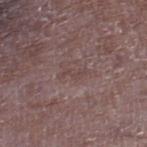Clinical impression: Captured during whole-body skin photography for melanoma surveillance; the lesion was not biopsied. Acquisition and patient details: This is a white-light tile. A male patient, in their mid-60s. A region of skin cropped from a whole-body photographic capture, roughly 15 mm wide. The lesion is located on the right lower leg. Measured at roughly 3 mm in maximum diameter.